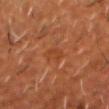Recorded during total-body skin imaging; not selected for excision or biopsy. The tile uses cross-polarized illumination. The lesion is located on the chest. A lesion tile, about 15 mm wide, cut from a 3D total-body photograph. Measured at roughly 2.5 mm in maximum diameter. A male subject, in their mid-50s.